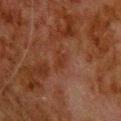Findings:
• follow-up: catalogued during a skin exam; not biopsied
• anatomic site: the upper back
• lesion size: about 2.5 mm
• tile lighting: cross-polarized
• TBP lesion metrics: a lesion color around L≈27 a*≈20 b*≈26 in CIELAB and a normalized border contrast of about 6; a nevus-likeness score of about 0/100 and a detector confidence of about 100 out of 100 that the crop contains a lesion
• subject: male, about 80 years old
• image source: ~15 mm tile from a whole-body skin photo